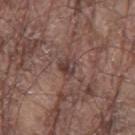The total-body-photography lesion software estimated a lesion color around L≈39 a*≈18 b*≈20 in CIELAB and a lesion-to-skin contrast of about 7.5 (normalized; higher = more distinct). The software also gave a border-irregularity index near 3/10 and a color-variation rating of about 4.5/10. The analysis additionally found a nevus-likeness score of about 0/100 and a detector confidence of about 55 out of 100 that the crop contains a lesion.
On the left thigh.
A male subject, aged around 80.
Imaged with white-light lighting.
A roughly 15 mm field-of-view crop from a total-body skin photograph.